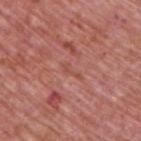Findings:
• workup — no biopsy performed (imaged during a skin exam)
• TBP lesion metrics — a lesion area of about 2 mm², an outline eccentricity of about 0.95 (0 = round, 1 = elongated), and a shape-asymmetry score of about 0.5 (0 = symmetric); an average lesion color of about L≈51 a*≈28 b*≈29 (CIELAB) and a lesion–skin lightness drop of about 5; a lesion-detection confidence of about 70/100
• diameter — ≈2.5 mm
• patient — male, aged around 75
• site — the upper back
• acquisition — ~15 mm tile from a whole-body skin photo